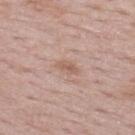workup = total-body-photography surveillance lesion; no biopsy
size = ≈3 mm
patient = female, approximately 50 years of age
imaging modality = 15 mm crop, total-body photography
image-analysis metrics = a border-irregularity rating of about 3/10, internal color variation of about 1 on a 0–10 scale, and a peripheral color-asymmetry measure near 0.5; a nevus-likeness score of about 0/100 and a detector confidence of about 100 out of 100 that the crop contains a lesion
site = the upper back